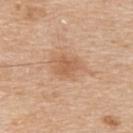{
  "lesion_size": {
    "long_diameter_mm_approx": 3.5
  },
  "automated_metrics": {
    "cielab_L": 59,
    "cielab_a": 21,
    "cielab_b": 35,
    "vs_skin_darker_L": 8.0,
    "vs_skin_contrast_norm": 6.0,
    "border_irregularity_0_10": 2.5,
    "color_variation_0_10": 2.0
  },
  "patient": {
    "sex": "male",
    "age_approx": 60
  },
  "image": {
    "source": "total-body photography crop",
    "field_of_view_mm": 15
  },
  "lighting": "white-light",
  "site": "upper back"
}